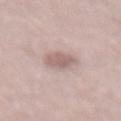Clinical impression: Captured during whole-body skin photography for melanoma surveillance; the lesion was not biopsied. Context: This is a white-light tile. A male subject aged around 55. A 15 mm close-up tile from a total-body photography series done for melanoma screening. The recorded lesion diameter is about 3.5 mm.